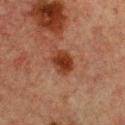{"biopsy_status": "not biopsied; imaged during a skin examination", "image": {"source": "total-body photography crop", "field_of_view_mm": 15}, "automated_metrics": {"area_mm2_approx": 7.5, "shape_asymmetry": 0.15, "cielab_L": 31, "cielab_a": 22, "cielab_b": 28, "vs_skin_darker_L": 10.0, "vs_skin_contrast_norm": 10.0, "border_irregularity_0_10": 1.5, "color_variation_0_10": 3.5, "peripheral_color_asymmetry": 1.0, "nevus_likeness_0_100": 95, "lesion_detection_confidence_0_100": 100}, "lesion_size": {"long_diameter_mm_approx": 3.5}, "site": "chest", "patient": {"sex": "female", "age_approx": 55}}